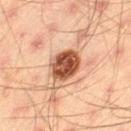follow-up = total-body-photography surveillance lesion; no biopsy | image = ~15 mm tile from a whole-body skin photo | patient = male, aged approximately 45 | size = ~4 mm (longest diameter) | image-analysis metrics = an area of roughly 10 mm², a shape eccentricity near 0.4, and a symmetry-axis asymmetry near 0.15; border irregularity of about 1.5 on a 0–10 scale, internal color variation of about 5.5 on a 0–10 scale, and a peripheral color-asymmetry measure near 2; a nevus-likeness score of about 90/100 and lesion-presence confidence of about 100/100 | site = the left thigh | lighting = cross-polarized.